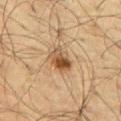Part of a total-body skin-imaging series; this lesion was reviewed on a skin check and was not flagged for biopsy. This is a cross-polarized tile. A male patient, approximately 65 years of age. A 15 mm crop from a total-body photograph taken for skin-cancer surveillance. The lesion is on the abdomen.